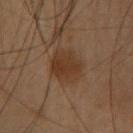Q: Was this lesion biopsied?
A: imaged on a skin check; not biopsied
Q: What is the anatomic site?
A: the head or neck
Q: What is the imaging modality?
A: ~15 mm tile from a whole-body skin photo
Q: Who is the patient?
A: female, approximately 55 years of age
Q: Illumination type?
A: cross-polarized
Q: Automated lesion metrics?
A: an average lesion color of about L≈29 a*≈15 b*≈25 (CIELAB), about 7 CIELAB-L* units darker than the surrounding skin, and a normalized border contrast of about 7.5; a within-lesion color-variation index near 2.5/10 and radial color variation of about 1; a nevus-likeness score of about 60/100 and a lesion-detection confidence of about 100/100
Q: What is the lesion's diameter?
A: about 4 mm